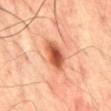notes: catalogued during a skin exam; not biopsied
body site: the front of the torso
lighting: cross-polarized illumination
imaging modality: ~15 mm crop, total-body skin-cancer survey
size: ~4 mm (longest diameter)
subject: male, aged approximately 70